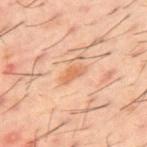This lesion was catalogued during total-body skin photography and was not selected for biopsy. The tile uses cross-polarized illumination. A close-up tile cropped from a whole-body skin photograph, about 15 mm across. The lesion is on the mid back. The patient is a male aged 58 to 62.